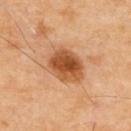Notes:
– notes: total-body-photography surveillance lesion; no biopsy
– subject: male, roughly 70 years of age
– image source: ~15 mm crop, total-body skin-cancer survey
– anatomic site: the upper back